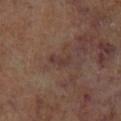Findings:
• workup · catalogued during a skin exam; not biopsied
• size · ~2.5 mm (longest diameter)
• anatomic site · the left lower leg
• image · total-body-photography crop, ~15 mm field of view
• TBP lesion metrics · a mean CIELAB color near L≈34 a*≈17 b*≈20, a lesion–skin lightness drop of about 5, and a normalized lesion–skin contrast near 6; a nevus-likeness score of about 0/100 and a lesion-detection confidence of about 100/100
• patient · male, in their 70s
• lighting · cross-polarized